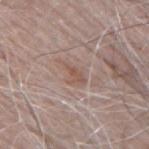{
  "biopsy_status": "not biopsied; imaged during a skin examination",
  "lighting": "white-light",
  "image": {
    "source": "total-body photography crop",
    "field_of_view_mm": 15
  },
  "lesion_size": {
    "long_diameter_mm_approx": 3.5
  },
  "patient": {
    "sex": "male",
    "age_approx": 65
  },
  "site": "upper back"
}